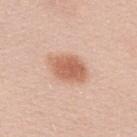Findings:
* biopsy status — no biopsy performed (imaged during a skin exam)
* image source — ~15 mm tile from a whole-body skin photo
* body site — the upper back
* lighting — white-light
* lesion size — ~4.5 mm (longest diameter)
* subject — male, approximately 25 years of age